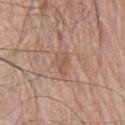This lesion was catalogued during total-body skin photography and was not selected for biopsy.
The tile uses white-light illumination.
A male patient, about 80 years old.
The recorded lesion diameter is about 2.5 mm.
Located on the chest.
A region of skin cropped from a whole-body photographic capture, roughly 15 mm wide.
The total-body-photography lesion software estimated a mean CIELAB color near L≈54 a*≈19 b*≈30, a lesion–skin lightness drop of about 6, and a lesion-to-skin contrast of about 5.5 (normalized; higher = more distinct). The analysis additionally found a border-irregularity index near 4.5/10 and a color-variation rating of about 1.5/10. The software also gave a nevus-likeness score of about 0/100 and lesion-presence confidence of about 90/100.